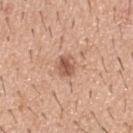Imaged during a routine full-body skin examination; the lesion was not biopsied and no histopathology is available. A roughly 15 mm field-of-view crop from a total-body skin photograph. The tile uses white-light illumination. The subject is a male aged 38 to 42. The lesion is on the upper back. Measured at roughly 3 mm in maximum diameter.